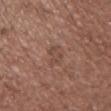workup — imaged on a skin check; not biopsied
body site — the chest
subject — male, aged 58–62
automated metrics — a footprint of about 5.5 mm²; a lesion color around L≈46 a*≈19 b*≈25 in CIELAB, roughly 6 lightness units darker than nearby skin, and a normalized lesion–skin contrast near 4.5; a border-irregularity rating of about 2.5/10 and a peripheral color-asymmetry measure near 0.5; a classifier nevus-likeness of about 0/100
lesion diameter — ~3 mm (longest diameter)
image source — 15 mm crop, total-body photography